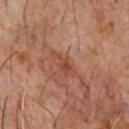Q: How was the tile lit?
A: cross-polarized illumination
Q: Patient demographics?
A: male, aged 58–62
Q: What is the imaging modality?
A: 15 mm crop, total-body photography
Q: Lesion location?
A: the chest
Q: Automated lesion metrics?
A: a footprint of about 4.5 mm², a shape eccentricity near 0.9, and a symmetry-axis asymmetry near 0.5; an automated nevus-likeness rating near 0 out of 100 and lesion-presence confidence of about 95/100